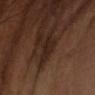Case summary:
- notes: total-body-photography surveillance lesion; no biopsy
- imaging modality: ~15 mm crop, total-body skin-cancer survey
- diameter: ~3.5 mm (longest diameter)
- patient: male, aged 63–67
- location: the arm
- lighting: cross-polarized
- automated lesion analysis: an area of roughly 6 mm², an outline eccentricity of about 0.8 (0 = round, 1 = elongated), and two-axis asymmetry of about 0.3; an automated nevus-likeness rating near 0 out of 100 and lesion-presence confidence of about 85/100